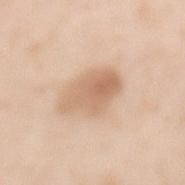<case>
  <biopsy_status>not biopsied; imaged during a skin examination</biopsy_status>
  <image>
    <source>total-body photography crop</source>
    <field_of_view_mm>15</field_of_view_mm>
  </image>
  <site>mid back</site>
  <lesion_size>
    <long_diameter_mm_approx>5.0</long_diameter_mm_approx>
  </lesion_size>
  <patient>
    <sex>female</sex>
    <age_approx>40</age_approx>
  </patient>
  <lighting>white-light</lighting>
</case>